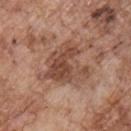biopsy status: imaged on a skin check; not biopsied | patient: male, aged around 70 | location: the chest | acquisition: ~15 mm tile from a whole-body skin photo | tile lighting: white-light illumination.